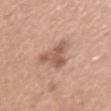- image source — ~15 mm tile from a whole-body skin photo
- tile lighting — white-light
- patient — female, aged 48–52
- location — the left upper arm